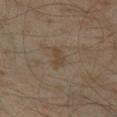Impression:
Imaged during a routine full-body skin examination; the lesion was not biopsied and no histopathology is available.
Background:
A region of skin cropped from a whole-body photographic capture, roughly 15 mm wide. The total-body-photography lesion software estimated an outline eccentricity of about 0.75 (0 = round, 1 = elongated) and a shape-asymmetry score of about 0.3 (0 = symmetric). The software also gave a border-irregularity index near 3/10, internal color variation of about 1 on a 0–10 scale, and peripheral color asymmetry of about 0.5. A male subject, aged 58 to 62. From the left thigh. About 3 mm across.